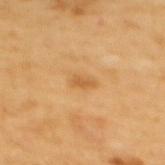notes — catalogued during a skin exam; not biopsied
patient — female, aged approximately 55
diameter — ~2.5 mm (longest diameter)
image — 15 mm crop, total-body photography
automated metrics — a footprint of about 2.5 mm²; a lesion–skin lightness drop of about 9 and a normalized border contrast of about 6; a classifier nevus-likeness of about 5/100 and a detector confidence of about 100 out of 100 that the crop contains a lesion
location — the upper back